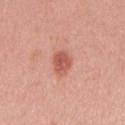Case summary:
* follow-up: catalogued during a skin exam; not biopsied
* image: total-body-photography crop, ~15 mm field of view
* subject: female, aged approximately 25
* body site: the right upper arm